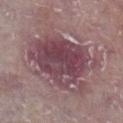Assessment:
Imaged during a routine full-body skin examination; the lesion was not biopsied and no histopathology is available.
Acquisition and patient details:
A 15 mm crop from a total-body photograph taken for skin-cancer surveillance. Automated tile analysis of the lesion measured an area of roughly 38 mm², an outline eccentricity of about 0.5 (0 = round, 1 = elongated), and two-axis asymmetry of about 0.3. Located on the right lower leg. A male subject, approximately 75 years of age.